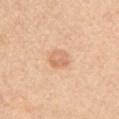notes: total-body-photography surveillance lesion; no biopsy
diameter: about 2.5 mm
automated lesion analysis: a footprint of about 5.5 mm², a shape eccentricity near 0.5, and two-axis asymmetry of about 0.15; a nevus-likeness score of about 10/100 and lesion-presence confidence of about 100/100
acquisition: 15 mm crop, total-body photography
lighting: white-light
site: the left upper arm
subject: male, aged around 65